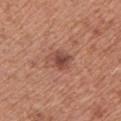Part of a total-body skin-imaging series; this lesion was reviewed on a skin check and was not flagged for biopsy. A close-up tile cropped from a whole-body skin photograph, about 15 mm across. About 3.5 mm across. The tile uses white-light illumination. A male subject, approximately 75 years of age. Located on the chest.